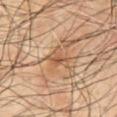Located on the front of the torso. A male patient, aged around 65. A 15 mm close-up tile from a total-body photography series done for melanoma screening.